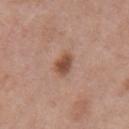biopsy status = no biopsy performed (imaged during a skin exam)
image source = ~15 mm tile from a whole-body skin photo
patient = female, aged 38–42
automated lesion analysis = a classifier nevus-likeness of about 95/100 and a detector confidence of about 100 out of 100 that the crop contains a lesion
site = the left upper arm